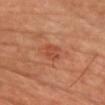{
  "biopsy_status": "not biopsied; imaged during a skin examination",
  "automated_metrics": {
    "border_irregularity_0_10": 2.5,
    "color_variation_0_10": 2.5,
    "peripheral_color_asymmetry": 1.0,
    "nevus_likeness_0_100": 5,
    "lesion_detection_confidence_0_100": 100
  },
  "patient": {
    "sex": "male",
    "age_approx": 65
  },
  "image": {
    "source": "total-body photography crop",
    "field_of_view_mm": 15
  },
  "lesion_size": {
    "long_diameter_mm_approx": 2.5
  },
  "lighting": "cross-polarized",
  "site": "chest"
}